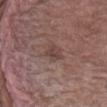Part of a total-body skin-imaging series; this lesion was reviewed on a skin check and was not flagged for biopsy. A 15 mm crop from a total-body photograph taken for skin-cancer surveillance. Captured under white-light illumination. A male subject in their mid-50s.